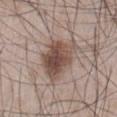{
  "biopsy_status": "not biopsied; imaged during a skin examination",
  "patient": {
    "sex": "male",
    "age_approx": 50
  },
  "lesion_size": {
    "long_diameter_mm_approx": 6.0
  },
  "lighting": "white-light",
  "image": {
    "source": "total-body photography crop",
    "field_of_view_mm": 15
  },
  "automated_metrics": {
    "eccentricity": 0.75,
    "shape_asymmetry": 0.3,
    "border_irregularity_0_10": 3.0,
    "color_variation_0_10": 6.5,
    "peripheral_color_asymmetry": 2.0,
    "nevus_likeness_0_100": 95,
    "lesion_detection_confidence_0_100": 100
  },
  "site": "abdomen"
}